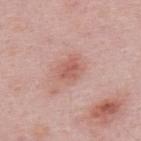Notes:
- follow-up — catalogued during a skin exam; not biopsied
- lesion size — ~2.5 mm (longest diameter)
- subject — male, aged approximately 50
- acquisition — ~15 mm tile from a whole-body skin photo
- image-analysis metrics — an average lesion color of about L≈57 a*≈27 b*≈26 (CIELAB), a lesion–skin lightness drop of about 9, and a normalized lesion–skin contrast near 6
- site — the upper back
- lighting — white-light illumination